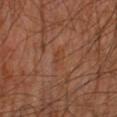Impression:
The lesion was tiled from a total-body skin photograph and was not biopsied.
Background:
Located on the right leg. A male subject, aged around 60. A close-up tile cropped from a whole-body skin photograph, about 15 mm across. About 2.5 mm across. Captured under cross-polarized illumination.